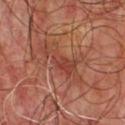{
  "automated_metrics": {
    "area_mm2_approx": 12.0,
    "eccentricity": 0.9,
    "shape_asymmetry": 0.45,
    "border_irregularity_0_10": 8.5,
    "color_variation_0_10": 3.5,
    "peripheral_color_asymmetry": 1.0,
    "nevus_likeness_0_100": 0,
    "lesion_detection_confidence_0_100": 85
  },
  "lesion_size": {
    "long_diameter_mm_approx": 6.5
  },
  "patient": {
    "sex": "male",
    "age_approx": 55
  },
  "site": "chest",
  "image": {
    "source": "total-body photography crop",
    "field_of_view_mm": 15
  },
  "lighting": "cross-polarized"
}